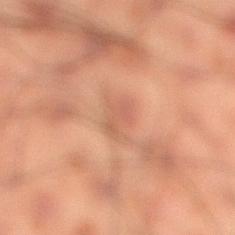Captured during whole-body skin photography for melanoma surveillance; the lesion was not biopsied. Longest diameter approximately 2.5 mm. From the leg. A 15 mm crop from a total-body photograph taken for skin-cancer surveillance. The total-body-photography lesion software estimated a border-irregularity index near 3.5/10, a color-variation rating of about 2/10, and radial color variation of about 0.5. The software also gave a classifier nevus-likeness of about 0/100 and a lesion-detection confidence of about 100/100. A male patient, approximately 50 years of age. The tile uses cross-polarized illumination.